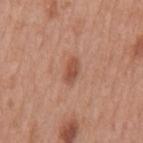biopsy status: imaged on a skin check; not biopsied | subject: male, aged 68 to 72 | location: the mid back | automated metrics: a lesion area of about 4.5 mm², an eccentricity of roughly 0.85, and two-axis asymmetry of about 0.25; border irregularity of about 2.5 on a 0–10 scale, a color-variation rating of about 2.5/10, and a peripheral color-asymmetry measure near 0.5; a classifier nevus-likeness of about 75/100 | tile lighting: white-light illumination | acquisition: total-body-photography crop, ~15 mm field of view | lesion size: about 3 mm.